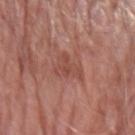Notes:
– patient: male, aged 78–82
– lighting: white-light
– lesion diameter: about 3.5 mm
– location: the left forearm
– image: 15 mm crop, total-body photography